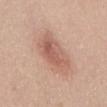- notes — imaged on a skin check; not biopsied
- patient — female, aged 43–47
- location — the abdomen
- illumination — white-light
- diameter — about 6 mm
- image — ~15 mm crop, total-body skin-cancer survey
- TBP lesion metrics — a lesion area of about 17 mm², an outline eccentricity of about 0.85 (0 = round, 1 = elongated), and a symmetry-axis asymmetry near 0.2; a lesion color around L≈59 a*≈22 b*≈28 in CIELAB, a lesion–skin lightness drop of about 10, and a normalized lesion–skin contrast near 6.5; internal color variation of about 5 on a 0–10 scale and radial color variation of about 1.5